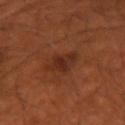workup: no biopsy performed (imaged during a skin exam); location: the left arm; image source: 15 mm crop, total-body photography; patient: male, aged around 50; tile lighting: cross-polarized.